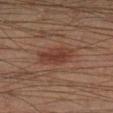Q: Was this lesion biopsied?
A: catalogued during a skin exam; not biopsied
Q: Who is the patient?
A: male, about 40 years old
Q: Lesion location?
A: the right lower leg
Q: Automated lesion metrics?
A: a footprint of about 9 mm², an eccentricity of roughly 0.85, and two-axis asymmetry of about 0.2; a border-irregularity index near 2/10
Q: What is the lesion's diameter?
A: ~4.5 mm (longest diameter)
Q: How was this image acquired?
A: ~15 mm crop, total-body skin-cancer survey
Q: What lighting was used for the tile?
A: cross-polarized